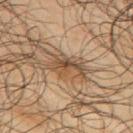| key | value |
|---|---|
| workup | no biopsy performed (imaged during a skin exam) |
| lighting | cross-polarized illumination |
| patient | male, approximately 65 years of age |
| body site | the upper back |
| image | ~15 mm tile from a whole-body skin photo |
| size | about 4 mm |